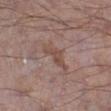Assessment:
No biopsy was performed on this lesion — it was imaged during a full skin examination and was not determined to be concerning.
Acquisition and patient details:
A male subject in their mid- to late 60s. From the right lower leg. The tile uses white-light illumination. A 15 mm crop from a total-body photograph taken for skin-cancer surveillance. About 5 mm across. An algorithmic analysis of the crop reported a lesion area of about 6 mm², an eccentricity of roughly 0.95, and a symmetry-axis asymmetry near 0.4. The analysis additionally found an average lesion color of about L≈48 a*≈18 b*≈24 (CIELAB), roughly 7 lightness units darker than nearby skin, and a normalized border contrast of about 6. The analysis additionally found a color-variation rating of about 1.5/10. The software also gave a nevus-likeness score of about 0/100 and a lesion-detection confidence of about 90/100.